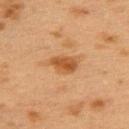Impression: Imaged during a routine full-body skin examination; the lesion was not biopsied and no histopathology is available. Image and clinical context: Cropped from a total-body skin-imaging series; the visible field is about 15 mm. Located on the upper back. A female subject aged 38 to 42.